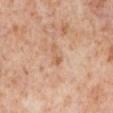Q: Where on the body is the lesion?
A: the right lower leg
Q: What did automated image analysis measure?
A: an outline eccentricity of about 0.95 (0 = round, 1 = elongated) and two-axis asymmetry of about 0.45; a normalized lesion–skin contrast near 6; a nevus-likeness score of about 0/100 and a lesion-detection confidence of about 100/100
Q: What is the lesion's diameter?
A: ≈3 mm
Q: What are the patient's age and sex?
A: female, aged 48 to 52
Q: What kind of image is this?
A: 15 mm crop, total-body photography
Q: Illumination type?
A: cross-polarized